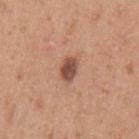No biopsy was performed on this lesion — it was imaged during a full skin examination and was not determined to be concerning.
Captured under white-light illumination.
The lesion is located on the left upper arm.
About 2.5 mm across.
Automated tile analysis of the lesion measured a border-irregularity index near 1/10, a within-lesion color-variation index near 2.5/10, and a peripheral color-asymmetry measure near 1. The software also gave a lesion-detection confidence of about 100/100.
A female subject about 40 years old.
A close-up tile cropped from a whole-body skin photograph, about 15 mm across.